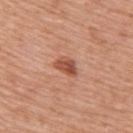The lesion was tiled from a total-body skin photograph and was not biopsied. This is a white-light tile. A close-up tile cropped from a whole-body skin photograph, about 15 mm across. The subject is a female about 55 years old. From the upper back. Approximately 3 mm at its widest. The total-body-photography lesion software estimated a lesion area of about 3.5 mm² and two-axis asymmetry of about 0.35. The analysis additionally found a mean CIELAB color near L≈51 a*≈26 b*≈33 and a lesion–skin lightness drop of about 13. The analysis additionally found border irregularity of about 3 on a 0–10 scale, internal color variation of about 4.5 on a 0–10 scale, and radial color variation of about 2.